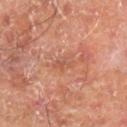Findings:
* image source — ~15 mm tile from a whole-body skin photo
* site — the right lower leg
* patient — male, approximately 70 years of age
* illumination — cross-polarized
* lesion diameter — ~2.5 mm (longest diameter)
* image-analysis metrics — a footprint of about 3.5 mm², an outline eccentricity of about 0.8 (0 = round, 1 = elongated), and a symmetry-axis asymmetry near 0.35; a lesion color around L≈54 a*≈25 b*≈31 in CIELAB, a lesion–skin lightness drop of about 7, and a lesion-to-skin contrast of about 4.5 (normalized; higher = more distinct); a peripheral color-asymmetry measure near 0; a classifier nevus-likeness of about 0/100 and lesion-presence confidence of about 100/100